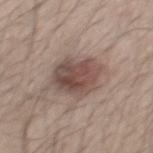A male patient roughly 40 years of age. This image is a 15 mm lesion crop taken from a total-body photograph. The lesion is on the mid back.